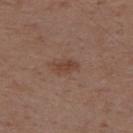Q: Is there a histopathology result?
A: imaged on a skin check; not biopsied
Q: Lesion location?
A: the right upper arm
Q: How was the tile lit?
A: white-light illumination
Q: What did automated image analysis measure?
A: a mean CIELAB color near L≈42 a*≈19 b*≈26, a lesion–skin lightness drop of about 7, and a normalized border contrast of about 6.5
Q: How large is the lesion?
A: about 2.5 mm
Q: What kind of image is this?
A: total-body-photography crop, ~15 mm field of view
Q: Patient demographics?
A: male, about 55 years old